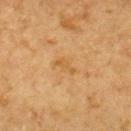Captured during whole-body skin photography for melanoma surveillance; the lesion was not biopsied.
Longest diameter approximately 3 mm.
From the upper back.
The tile uses cross-polarized illumination.
A male subject about 85 years old or older.
This image is a 15 mm lesion crop taken from a total-body photograph.